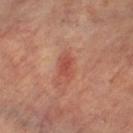Assessment: Recorded during total-body skin imaging; not selected for excision or biopsy. Background: An algorithmic analysis of the crop reported an automated nevus-likeness rating near 30 out of 100 and lesion-presence confidence of about 100/100. A roughly 15 mm field-of-view crop from a total-body skin photograph. About 3.5 mm across. Located on the right leg. A female subject approximately 65 years of age. Captured under cross-polarized illumination.